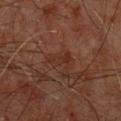Notes:
* notes: catalogued during a skin exam; not biopsied
* size: ≈3 mm
* location: the upper back
* patient: male, aged 58–62
* image source: total-body-photography crop, ~15 mm field of view
* tile lighting: cross-polarized illumination